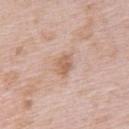Clinical impression: The lesion was photographed on a routine skin check and not biopsied; there is no pathology result. Acquisition and patient details: A female subject, in their mid-60s. Imaged with white-light lighting. A 15 mm close-up extracted from a 3D total-body photography capture. On the upper back. Automated image analysis of the tile measured a lesion area of about 4.5 mm² and two-axis asymmetry of about 0.25. The recorded lesion diameter is about 2.5 mm.